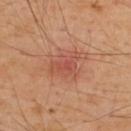This lesion was catalogued during total-body skin photography and was not selected for biopsy.
The lesion-visualizer software estimated a lesion area of about 8 mm², an outline eccentricity of about 0.7 (0 = round, 1 = elongated), and a shape-asymmetry score of about 0.4 (0 = symmetric).
The subject is a male aged 48 to 52.
The lesion's longest dimension is about 4 mm.
The lesion is located on the upper back.
A close-up tile cropped from a whole-body skin photograph, about 15 mm across.